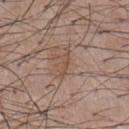– follow-up · imaged on a skin check; not biopsied
– imaging modality · ~15 mm tile from a whole-body skin photo
– patient · male, aged around 55
– location · the chest